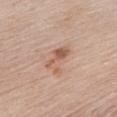<lesion>
  <biopsy_status>not biopsied; imaged during a skin examination</biopsy_status>
  <site>chest</site>
  <automated_metrics>
    <area_mm2_approx>7.0</area_mm2_approx>
    <eccentricity>0.85</eccentricity>
    <cielab_L>59</cielab_L>
    <cielab_a>21</cielab_a>
    <cielab_b>30</cielab_b>
    <vs_skin_darker_L>9.0</vs_skin_darker_L>
    <vs_skin_contrast_norm>6.5</vs_skin_contrast_norm>
    <lesion_detection_confidence_0_100>100</lesion_detection_confidence_0_100>
  </automated_metrics>
  <image>
    <source>total-body photography crop</source>
    <field_of_view_mm>15</field_of_view_mm>
  </image>
  <patient>
    <sex>female</sex>
    <age_approx>65</age_approx>
  </patient>
  <lesion_size>
    <long_diameter_mm_approx>4.0</long_diameter_mm_approx>
  </lesion_size>
  <lighting>white-light</lighting>
</lesion>